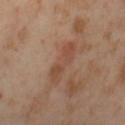Image and clinical context: Captured under cross-polarized illumination. The lesion's longest dimension is about 5 mm. From the left thigh. A 15 mm close-up tile from a total-body photography series done for melanoma screening. A female patient approximately 55 years of age.